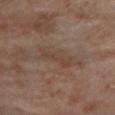A 15 mm close-up extracted from a 3D total-body photography capture. The lesion is on the left lower leg. A male subject aged 68 to 72.